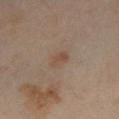No biopsy was performed on this lesion — it was imaged during a full skin examination and was not determined to be concerning.
A close-up tile cropped from a whole-body skin photograph, about 15 mm across.
Automated image analysis of the tile measured an area of roughly 4 mm² and two-axis asymmetry of about 0.35.
A male subject, in their mid- to late 60s.
Captured under cross-polarized illumination.
The lesion is on the left lower leg.